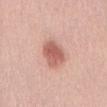Part of a total-body skin-imaging series; this lesion was reviewed on a skin check and was not flagged for biopsy. This image is a 15 mm lesion crop taken from a total-body photograph. The total-body-photography lesion software estimated an outline eccentricity of about 0.75 (0 = round, 1 = elongated) and a symmetry-axis asymmetry near 0.15. And it measured border irregularity of about 1.5 on a 0–10 scale, internal color variation of about 3.5 on a 0–10 scale, and a peripheral color-asymmetry measure near 1. Measured at roughly 4 mm in maximum diameter. The subject is a male in their 30s.